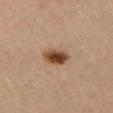notes: no biopsy performed (imaged during a skin exam); diameter: about 3 mm; acquisition: ~15 mm crop, total-body skin-cancer survey; subject: female, aged 43–47; site: the right upper arm.